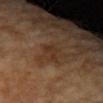follow-up: imaged on a skin check; not biopsied | tile lighting: cross-polarized illumination | site: the right forearm | imaging modality: total-body-photography crop, ~15 mm field of view | subject: female, roughly 70 years of age | lesion diameter: about 3 mm | TBP lesion metrics: a classifier nevus-likeness of about 0/100 and lesion-presence confidence of about 100/100.